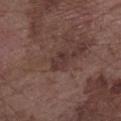Impression:
Imaged during a routine full-body skin examination; the lesion was not biopsied and no histopathology is available.
Clinical summary:
On the right forearm. Imaged with white-light lighting. About 2.5 mm across. A 15 mm close-up tile from a total-body photography series done for melanoma screening. A male subject, approximately 75 years of age. Automated image analysis of the tile measured a normalized lesion–skin contrast near 6.5.